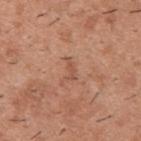Recorded during total-body skin imaging; not selected for excision or biopsy. Automated tile analysis of the lesion measured an area of roughly 2.5 mm² and an eccentricity of roughly 0.9. And it measured a mean CIELAB color near L≈53 a*≈22 b*≈31, about 7 CIELAB-L* units darker than the surrounding skin, and a normalized border contrast of about 5.5. The software also gave a nevus-likeness score of about 0/100 and lesion-presence confidence of about 100/100. About 2.5 mm across. Imaged with white-light lighting. A close-up tile cropped from a whole-body skin photograph, about 15 mm across. A male subject, about 40 years old. From the upper back.